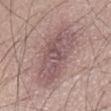Captured during whole-body skin photography for melanoma surveillance; the lesion was not biopsied. On the abdomen. Cropped from a whole-body photographic skin survey; the tile spans about 15 mm. A male patient about 50 years old. Captured under white-light illumination. The lesion-visualizer software estimated a footprint of about 28 mm², a shape eccentricity near 0.95, and two-axis asymmetry of about 0.2. The software also gave a border-irregularity index near 3.5/10, internal color variation of about 4.5 on a 0–10 scale, and radial color variation of about 1.5.